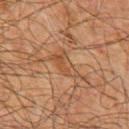Q: Was this lesion biopsied?
A: catalogued during a skin exam; not biopsied
Q: What lighting was used for the tile?
A: cross-polarized illumination
Q: What did automated image analysis measure?
A: a footprint of about 6 mm², an eccentricity of roughly 0.85, and a symmetry-axis asymmetry near 0.4
Q: Where on the body is the lesion?
A: the chest
Q: Who is the patient?
A: male, aged around 60
Q: What kind of image is this?
A: ~15 mm tile from a whole-body skin photo